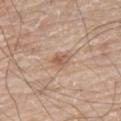This lesion was catalogued during total-body skin photography and was not selected for biopsy.
Captured under white-light illumination.
A male subject approximately 80 years of age.
On the left leg.
A roughly 15 mm field-of-view crop from a total-body skin photograph.
The lesion's longest dimension is about 2.5 mm.
The total-body-photography lesion software estimated an area of roughly 3 mm², an outline eccentricity of about 0.8 (0 = round, 1 = elongated), and a shape-asymmetry score of about 0.25 (0 = symmetric). It also reported about 10 CIELAB-L* units darker than the surrounding skin and a lesion-to-skin contrast of about 7 (normalized; higher = more distinct).